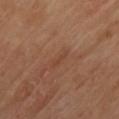Part of a total-body skin-imaging series; this lesion was reviewed on a skin check and was not flagged for biopsy. Located on the chest. A region of skin cropped from a whole-body photographic capture, roughly 15 mm wide.